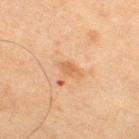This lesion was catalogued during total-body skin photography and was not selected for biopsy. A male patient, roughly 65 years of age. The total-body-photography lesion software estimated an eccentricity of roughly 0.85 and two-axis asymmetry of about 0.2. The software also gave border irregularity of about 2 on a 0–10 scale, a within-lesion color-variation index near 1/10, and a peripheral color-asymmetry measure near 0.5. And it measured a lesion-detection confidence of about 100/100. On the right thigh. This is a cross-polarized tile. A 15 mm crop from a total-body photograph taken for skin-cancer surveillance.